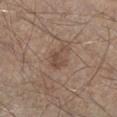biopsy status: no biopsy performed (imaged during a skin exam) | subject: male, aged 43 to 47 | image source: total-body-photography crop, ~15 mm field of view | location: the left lower leg.